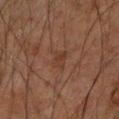Q: Was a biopsy performed?
A: imaged on a skin check; not biopsied
Q: Where on the body is the lesion?
A: the left arm
Q: Patient demographics?
A: male, approximately 60 years of age
Q: How was this image acquired?
A: total-body-photography crop, ~15 mm field of view